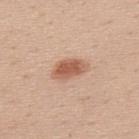The lesion was photographed on a routine skin check and not biopsied; there is no pathology result.
Imaged with white-light lighting.
Automated tile analysis of the lesion measured an eccentricity of roughly 0.85 and a symmetry-axis asymmetry near 0.2. The analysis additionally found a lesion color around L≈58 a*≈23 b*≈31 in CIELAB and a lesion-to-skin contrast of about 8 (normalized; higher = more distinct). The analysis additionally found a border-irregularity index near 2/10, a within-lesion color-variation index near 3.5/10, and a peripheral color-asymmetry measure near 1.
This image is a 15 mm lesion crop taken from a total-body photograph.
About 4.5 mm across.
The lesion is located on the upper back.
A male subject, roughly 40 years of age.